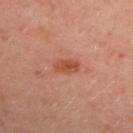The lesion was tiled from a total-body skin photograph and was not biopsied. From the chest. Cropped from a whole-body photographic skin survey; the tile spans about 15 mm. A female patient roughly 50 years of age. Measured at roughly 3 mm in maximum diameter.